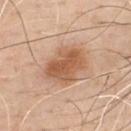Notes:
• workup · total-body-photography surveillance lesion; no biopsy
• patient · male, aged around 50
• image-analysis metrics · a lesion-to-skin contrast of about 8.5 (normalized; higher = more distinct); a border-irregularity index near 2.5/10 and a within-lesion color-variation index near 4/10; a nevus-likeness score of about 55/100
• lesion diameter · ≈5 mm
• anatomic site · the chest
• imaging modality · ~15 mm tile from a whole-body skin photo